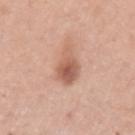Q: Was a biopsy performed?
A: imaged on a skin check; not biopsied
Q: What is the anatomic site?
A: the left upper arm
Q: Automated lesion metrics?
A: a mean CIELAB color near L≈59 a*≈22 b*≈30 and about 12 CIELAB-L* units darker than the surrounding skin
Q: How was this image acquired?
A: ~15 mm tile from a whole-body skin photo
Q: How was the tile lit?
A: white-light
Q: Patient demographics?
A: male, about 50 years old
Q: Lesion size?
A: ~5 mm (longest diameter)